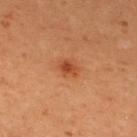Q: Was this lesion biopsied?
A: no biopsy performed (imaged during a skin exam)
Q: How was the tile lit?
A: cross-polarized
Q: What kind of image is this?
A: 15 mm crop, total-body photography
Q: Lesion location?
A: the upper back
Q: Lesion size?
A: ~2.5 mm (longest diameter)
Q: Automated lesion metrics?
A: a normalized border contrast of about 7.5; peripheral color asymmetry of about 1
Q: Patient demographics?
A: female, in their mid-30s